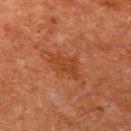Findings:
– follow-up · imaged on a skin check; not biopsied
– patient · male, aged 58 to 62
– image · ~15 mm crop, total-body skin-cancer survey
– location · the arm
– lesion size · ≈4 mm